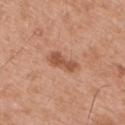Q: Was this lesion biopsied?
A: catalogued during a skin exam; not biopsied
Q: What are the patient's age and sex?
A: male, in their mid- to late 50s
Q: What lighting was used for the tile?
A: white-light illumination
Q: What kind of image is this?
A: total-body-photography crop, ~15 mm field of view
Q: Lesion location?
A: the right upper arm